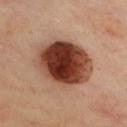Assessment: The lesion was photographed on a routine skin check and not biopsied; there is no pathology result. Context: From the chest. A male patient aged around 45. Cropped from a whole-body photographic skin survey; the tile spans about 15 mm.